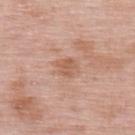This lesion was catalogued during total-body skin photography and was not selected for biopsy. The lesion is on the upper back. The patient is a female aged around 50. The tile uses white-light illumination. This image is a 15 mm lesion crop taken from a total-body photograph. About 2.5 mm across. The total-body-photography lesion software estimated an outline eccentricity of about 0.55 (0 = round, 1 = elongated) and two-axis asymmetry of about 0.25. The analysis additionally found a lesion color around L≈58 a*≈22 b*≈31 in CIELAB, a lesion–skin lightness drop of about 8, and a normalized border contrast of about 5.5. It also reported an automated nevus-likeness rating near 0 out of 100 and a lesion-detection confidence of about 100/100.